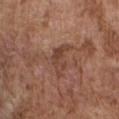Measured at roughly 4 mm in maximum diameter. Cropped from a whole-body photographic skin survey; the tile spans about 15 mm. From the chest. A male subject, in their mid- to late 70s.